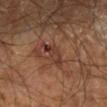{"biopsy_status": "not biopsied; imaged during a skin examination", "site": "left lower leg", "image": {"source": "total-body photography crop", "field_of_view_mm": 15}, "lesion_size": {"long_diameter_mm_approx": 4.0}, "lighting": "cross-polarized", "patient": {"age_approx": 65}, "automated_metrics": {"nevus_likeness_0_100": 0, "lesion_detection_confidence_0_100": 100}}